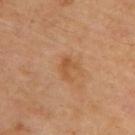Impression: This lesion was catalogued during total-body skin photography and was not selected for biopsy. Image and clinical context: The lesion is on the upper back. Cropped from a total-body skin-imaging series; the visible field is about 15 mm. Measured at roughly 2.5 mm in maximum diameter. The patient is a male aged 68 to 72. Captured under cross-polarized illumination.